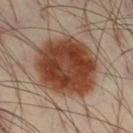  biopsy_status: not biopsied; imaged during a skin examination
  lighting: cross-polarized
  image:
    source: total-body photography crop
    field_of_view_mm: 15
  lesion_size:
    long_diameter_mm_approx: 7.0
  patient:
    sex: male
    age_approx: 40
  site: leg